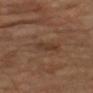Q: Was this lesion biopsied?
A: catalogued during a skin exam; not biopsied
Q: What kind of image is this?
A: ~15 mm tile from a whole-body skin photo
Q: Patient demographics?
A: male, roughly 65 years of age
Q: What is the anatomic site?
A: the arm
Q: What is the lesion's diameter?
A: ≈3.5 mm
Q: How was the tile lit?
A: cross-polarized illumination